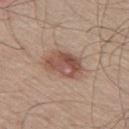Clinical impression: The lesion was tiled from a total-body skin photograph and was not biopsied. Context: From the leg. About 5 mm across. The subject is a male aged approximately 60. This image is a 15 mm lesion crop taken from a total-body photograph.